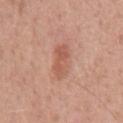Part of a total-body skin-imaging series; this lesion was reviewed on a skin check and was not flagged for biopsy. Measured at roughly 4 mm in maximum diameter. A male subject, aged approximately 50. A lesion tile, about 15 mm wide, cut from a 3D total-body photograph. On the chest. This is a white-light tile.